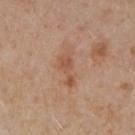{
  "biopsy_status": "not biopsied; imaged during a skin examination",
  "site": "left arm",
  "lesion_size": {
    "long_diameter_mm_approx": 4.0
  },
  "image": {
    "source": "total-body photography crop",
    "field_of_view_mm": 15
  },
  "automated_metrics": {
    "shape_asymmetry": 0.5,
    "nevus_likeness_0_100": 0,
    "lesion_detection_confidence_0_100": 100
  },
  "patient": {
    "sex": "female",
    "age_approx": 40
  }
}